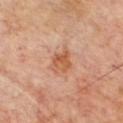• notes — imaged on a skin check; not biopsied
• diameter — about 3 mm
• image — total-body-photography crop, ~15 mm field of view
• patient — male, approximately 70 years of age
• anatomic site — the chest
• TBP lesion metrics — a border-irregularity rating of about 3/10, a within-lesion color-variation index near 4/10, and peripheral color asymmetry of about 1.5
• tile lighting — cross-polarized